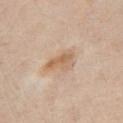<case>
  <biopsy_status>not biopsied; imaged during a skin examination</biopsy_status>
  <patient>
    <sex>male</sex>
    <age_approx>75</age_approx>
  </patient>
  <lesion_size>
    <long_diameter_mm_approx>3.5</long_diameter_mm_approx>
  </lesion_size>
  <image>
    <source>total-body photography crop</source>
    <field_of_view_mm>15</field_of_view_mm>
  </image>
  <automated_metrics>
    <cielab_L>63</cielab_L>
    <cielab_a>17</cielab_a>
    <cielab_b>33</cielab_b>
    <vs_skin_darker_L>9.0</vs_skin_darker_L>
    <vs_skin_contrast_norm>7.0</vs_skin_contrast_norm>
    <border_irregularity_0_10>4.0</border_irregularity_0_10>
    <lesion_detection_confidence_0_100>100</lesion_detection_confidence_0_100>
  </automated_metrics>
  <site>chest</site>
</case>